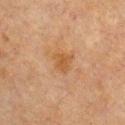Q: Is there a histopathology result?
A: catalogued during a skin exam; not biopsied
Q: What is the imaging modality?
A: 15 mm crop, total-body photography
Q: What did automated image analysis measure?
A: an eccentricity of roughly 0.5; a lesion color around L≈44 a*≈18 b*≈33 in CIELAB and a lesion–skin lightness drop of about 6; a lesion-detection confidence of about 100/100
Q: How large is the lesion?
A: ≈2.5 mm
Q: Where on the body is the lesion?
A: the chest
Q: Patient demographics?
A: female, roughly 55 years of age
Q: Illumination type?
A: cross-polarized illumination